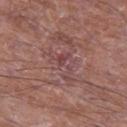follow-up — no biopsy performed (imaged during a skin exam) | acquisition — ~15 mm crop, total-body skin-cancer survey | lesion size — ~4.5 mm (longest diameter) | tile lighting — white-light | anatomic site — the left thigh | automated metrics — an area of roughly 7 mm², an outline eccentricity of about 0.8 (0 = round, 1 = elongated), and a shape-asymmetry score of about 0.6 (0 = symmetric); a mean CIELAB color near L≈46 a*≈22 b*≈19, roughly 6 lightness units darker than nearby skin, and a normalized lesion–skin contrast near 5 | patient — male, in their mid-60s.